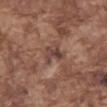{"biopsy_status": "not biopsied; imaged during a skin examination", "patient": {"sex": "male", "age_approx": 75}, "site": "abdomen", "image": {"source": "total-body photography crop", "field_of_view_mm": 15}}